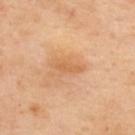Assessment: The lesion was tiled from a total-body skin photograph and was not biopsied. Acquisition and patient details: On the back. A lesion tile, about 15 mm wide, cut from a 3D total-body photograph. The patient is a female about 40 years old.